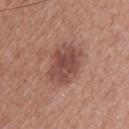| field | value |
|---|---|
| notes | total-body-photography surveillance lesion; no biopsy |
| lighting | white-light illumination |
| acquisition | ~15 mm tile from a whole-body skin photo |
| body site | the upper back |
| TBP lesion metrics | a footprint of about 15 mm² and a shape eccentricity near 0.7; a border-irregularity rating of about 2.5/10, a color-variation rating of about 3.5/10, and peripheral color asymmetry of about 1 |
| patient | female, aged 38–42 |
| diameter | ≈5 mm |